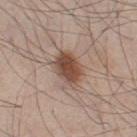workup: total-body-photography surveillance lesion; no biopsy
patient: male, aged approximately 45
image source: 15 mm crop, total-body photography
diameter: about 4 mm
image-analysis metrics: a border-irregularity index near 1.5/10, a within-lesion color-variation index near 4.5/10, and radial color variation of about 1.5; an automated nevus-likeness rating near 100 out of 100 and a detector confidence of about 100 out of 100 that the crop contains a lesion
location: the left thigh
tile lighting: white-light illumination